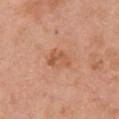follow-up = imaged on a skin check; not biopsied
subject = female, approximately 60 years of age
image-analysis metrics = an automated nevus-likeness rating near 5 out of 100 and a detector confidence of about 100 out of 100 that the crop contains a lesion
lighting = white-light
anatomic site = the chest
imaging modality = ~15 mm crop, total-body skin-cancer survey